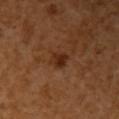Clinical impression: Imaged during a routine full-body skin examination; the lesion was not biopsied and no histopathology is available. Background: Captured under cross-polarized illumination. On the right upper arm. Approximately 3 mm at its widest. A female subject, in their mid-50s. The total-body-photography lesion software estimated a lesion–skin lightness drop of about 9 and a normalized border contrast of about 8.5. The analysis additionally found a color-variation rating of about 3.5/10 and peripheral color asymmetry of about 1.5. The analysis additionally found an automated nevus-likeness rating near 80 out of 100 and a detector confidence of about 100 out of 100 that the crop contains a lesion. A 15 mm crop from a total-body photograph taken for skin-cancer surveillance.